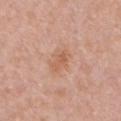The lesion was photographed on a routine skin check and not biopsied; there is no pathology result. The lesion is on the front of the torso. The lesion-visualizer software estimated a within-lesion color-variation index near 3/10. Approximately 3.5 mm at its widest. A 15 mm crop from a total-body photograph taken for skin-cancer surveillance. A female patient, approximately 40 years of age. This is a white-light tile.